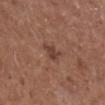No biopsy was performed on this lesion — it was imaged during a full skin examination and was not determined to be concerning. The lesion is on the right lower leg. The subject is a male aged 73 to 77. A lesion tile, about 15 mm wide, cut from a 3D total-body photograph. The tile uses white-light illumination. The lesion-visualizer software estimated a lesion area of about 4.5 mm², an eccentricity of roughly 0.55, and a shape-asymmetry score of about 0.3 (0 = symmetric). It also reported an average lesion color of about L≈41 a*≈20 b*≈25 (CIELAB), a lesion–skin lightness drop of about 8, and a normalized lesion–skin contrast near 7. The analysis additionally found border irregularity of about 3.5 on a 0–10 scale and a peripheral color-asymmetry measure near 0.5. The lesion's longest dimension is about 2.5 mm.